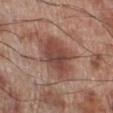patient: male, roughly 70 years of age
image-analysis metrics: a footprint of about 18 mm², a shape eccentricity near 0.7, and a shape-asymmetry score of about 0.25 (0 = symmetric); an automated nevus-likeness rating near 40 out of 100 and a lesion-detection confidence of about 100/100
body site: the right lower leg
size: about 6 mm
image: ~15 mm crop, total-body skin-cancer survey